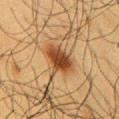{
  "biopsy_status": "not biopsied; imaged during a skin examination",
  "automated_metrics": {
    "eccentricity": 0.7,
    "shape_asymmetry": 0.2,
    "cielab_L": 36,
    "cielab_a": 19,
    "cielab_b": 31,
    "vs_skin_darker_L": 12.0,
    "vs_skin_contrast_norm": 10.5,
    "border_irregularity_0_10": 2.5,
    "color_variation_0_10": 4.5,
    "peripheral_color_asymmetry": 1.0,
    "nevus_likeness_0_100": 100,
    "lesion_detection_confidence_0_100": 100
  },
  "lighting": "cross-polarized",
  "site": "chest",
  "patient": {
    "sex": "male",
    "age_approx": 60
  },
  "image": {
    "source": "total-body photography crop",
    "field_of_view_mm": 15
  },
  "lesion_size": {
    "long_diameter_mm_approx": 4.0
  }
}